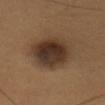{"biopsy_status": "not biopsied; imaged during a skin examination", "image": {"source": "total-body photography crop", "field_of_view_mm": 15}, "lesion_size": {"long_diameter_mm_approx": 6.0}, "site": "head or neck", "lighting": "cross-polarized", "patient": {"sex": "female", "age_approx": 30}}